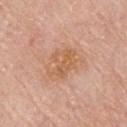From the upper back. Imaged with white-light lighting. A male subject aged around 70. The lesion's longest dimension is about 5 mm. A lesion tile, about 15 mm wide, cut from a 3D total-body photograph.